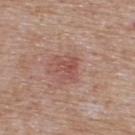Image and clinical context: Measured at roughly 2.5 mm in maximum diameter. Captured under white-light illumination. From the upper back. A male subject about 75 years old. A roughly 15 mm field-of-view crop from a total-body skin photograph.